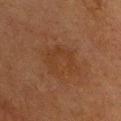  biopsy_status: not biopsied; imaged during a skin examination
  image:
    source: total-body photography crop
    field_of_view_mm: 15
  site: chest
  patient:
    sex: male
    age_approx: 75